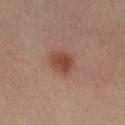A female patient, approximately 40 years of age. From the right thigh. A roughly 15 mm field-of-view crop from a total-body skin photograph. This is a cross-polarized tile. Automated image analysis of the tile measured a border-irregularity rating of about 1/10, internal color variation of about 3 on a 0–10 scale, and radial color variation of about 1.